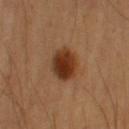lesion size: ~4 mm (longest diameter)
illumination: cross-polarized illumination
body site: the arm
acquisition: total-body-photography crop, ~15 mm field of view
image-analysis metrics: an area of roughly 11 mm², an outline eccentricity of about 0.55 (0 = round, 1 = elongated), and a shape-asymmetry score of about 0.1 (0 = symmetric); internal color variation of about 5 on a 0–10 scale and a peripheral color-asymmetry measure near 1.5
subject: male, approximately 70 years of age